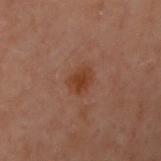| field | value |
|---|---|
| biopsy status | catalogued during a skin exam; not biopsied |
| illumination | cross-polarized |
| acquisition | 15 mm crop, total-body photography |
| location | the left arm |
| subject | female, about 60 years old |